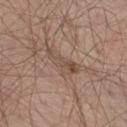Q: Was a biopsy performed?
A: total-body-photography surveillance lesion; no biopsy
Q: What is the anatomic site?
A: the right thigh
Q: What is the lesion's diameter?
A: ≈4.5 mm
Q: Patient demographics?
A: male, in their mid-60s
Q: What kind of image is this?
A: total-body-photography crop, ~15 mm field of view